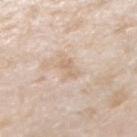<record>
<biopsy_status>not biopsied; imaged during a skin examination</biopsy_status>
<lesion_size>
  <long_diameter_mm_approx>3.0</long_diameter_mm_approx>
</lesion_size>
<site>arm</site>
<image>
  <source>total-body photography crop</source>
  <field_of_view_mm>15</field_of_view_mm>
</image>
<patient>
  <sex>male</sex>
  <age_approx>45</age_approx>
</patient>
</record>